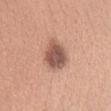Notes:
* imaging modality · ~15 mm crop, total-body skin-cancer survey
* tile lighting · white-light
* size · ~4.5 mm (longest diameter)
* automated metrics · a border-irregularity rating of about 2/10, internal color variation of about 5.5 on a 0–10 scale, and a peripheral color-asymmetry measure near 1.5; a classifier nevus-likeness of about 70/100 and lesion-presence confidence of about 100/100
* location · the head or neck
* subject · female, aged 28 to 32